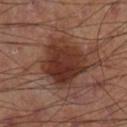Clinical impression:
Imaged during a routine full-body skin examination; the lesion was not biopsied and no histopathology is available.
Image and clinical context:
Measured at roughly 5.5 mm in maximum diameter. This is a cross-polarized tile. From the right lower leg. A close-up tile cropped from a whole-body skin photograph, about 15 mm across.